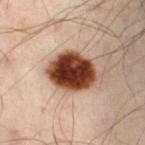Clinical impression: The lesion was tiled from a total-body skin photograph and was not biopsied. Background: Captured under cross-polarized illumination. On the lower back. Longest diameter approximately 5.5 mm. A 15 mm close-up extracted from a 3D total-body photography capture. An algorithmic analysis of the crop reported an eccentricity of roughly 0.6 and two-axis asymmetry of about 0.15. And it measured a mean CIELAB color near L≈40 a*≈24 b*≈31, roughly 28 lightness units darker than nearby skin, and a normalized lesion–skin contrast near 19. The analysis additionally found internal color variation of about 8 on a 0–10 scale and a peripheral color-asymmetry measure near 2.5. It also reported a classifier nevus-likeness of about 100/100 and lesion-presence confidence of about 100/100. The patient is a male aged 48 to 52.